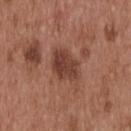Part of a total-body skin-imaging series; this lesion was reviewed on a skin check and was not flagged for biopsy. An algorithmic analysis of the crop reported an area of roughly 10 mm², an eccentricity of roughly 0.65, and two-axis asymmetry of about 0.25. And it measured a lesion-detection confidence of about 100/100. Captured under white-light illumination. The lesion is on the head or neck. The subject is a male in their mid- to late 50s. A 15 mm close-up extracted from a 3D total-body photography capture. The recorded lesion diameter is about 4 mm.